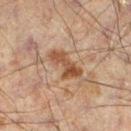  biopsy_status: not biopsied; imaged during a skin examination
  automated_metrics:
    eccentricity: 0.9
  lesion_size:
    long_diameter_mm_approx: 4.5
  image:
    source: total-body photography crop
    field_of_view_mm: 15
  patient:
    sex: male
    age_approx: 60
  site: left lower leg
  lighting: cross-polarized